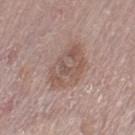Clinical impression: The lesion was photographed on a routine skin check and not biopsied; there is no pathology result. Context: Automated tile analysis of the lesion measured a lesion area of about 16 mm², an outline eccentricity of about 0.8 (0 = round, 1 = elongated), and a symmetry-axis asymmetry near 0.2. And it measured a lesion color around L≈54 a*≈17 b*≈23 in CIELAB, about 8 CIELAB-L* units darker than the surrounding skin, and a normalized border contrast of about 6. It also reported a color-variation rating of about 5.5/10 and a peripheral color-asymmetry measure near 2. It also reported a classifier nevus-likeness of about 0/100 and lesion-presence confidence of about 100/100. A female patient in their mid- to late 60s. A region of skin cropped from a whole-body photographic capture, roughly 15 mm wide. Measured at roughly 6 mm in maximum diameter. The lesion is on the left thigh.